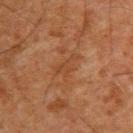Part of a total-body skin-imaging series; this lesion was reviewed on a skin check and was not flagged for biopsy.
The tile uses cross-polarized illumination.
An algorithmic analysis of the crop reported an average lesion color of about L≈35 a*≈20 b*≈29 (CIELAB), about 5 CIELAB-L* units darker than the surrounding skin, and a normalized border contrast of about 5. It also reported a nevus-likeness score of about 0/100 and a detector confidence of about 100 out of 100 that the crop contains a lesion.
A close-up tile cropped from a whole-body skin photograph, about 15 mm across.
On the upper back.
A male patient, aged approximately 65.